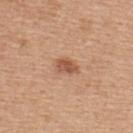Clinical impression: No biopsy was performed on this lesion — it was imaged during a full skin examination and was not determined to be concerning. Background: A region of skin cropped from a whole-body photographic capture, roughly 15 mm wide. Captured under white-light illumination. A female subject aged approximately 45. From the upper back. Approximately 2.5 mm at its widest.